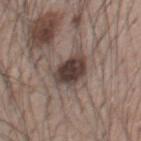No biopsy was performed on this lesion — it was imaged during a full skin examination and was not determined to be concerning.
Captured under white-light illumination.
From the chest.
A male subject aged 53 to 57.
Approximately 4 mm at its widest.
A 15 mm close-up extracted from a 3D total-body photography capture.